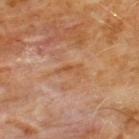This lesion was catalogued during total-body skin photography and was not selected for biopsy. A 15 mm close-up tile from a total-body photography series done for melanoma screening. The patient is a male approximately 60 years of age. On the chest.